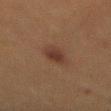Findings:
– biopsy status: imaged on a skin check; not biopsied
– image source: 15 mm crop, total-body photography
– subject: male, aged 48 to 52
– site: the mid back
– lesion diameter: ~3.5 mm (longest diameter)
– lighting: cross-polarized